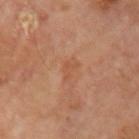Notes:
• diameter — about 2.5 mm
• subject — male, aged 68–72
• imaging modality — ~15 mm crop, total-body skin-cancer survey
• body site — the left upper arm